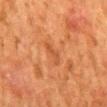Findings:
* workup — catalogued during a skin exam; not biopsied
* lesion diameter — about 3 mm
* image source — ~15 mm crop, total-body skin-cancer survey
* illumination — cross-polarized
* subject — female, aged 48 to 52
* site — the mid back